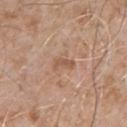<tbp_lesion>
  <lesion_size>
    <long_diameter_mm_approx>2.5</long_diameter_mm_approx>
  </lesion_size>
  <patient>
    <sex>male</sex>
    <age_approx>55</age_approx>
  </patient>
  <lighting>white-light</lighting>
  <image>
    <source>total-body photography crop</source>
    <field_of_view_mm>15</field_of_view_mm>
  </image>
  <site>chest</site>
</tbp_lesion>